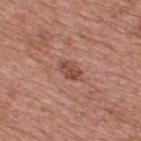Acquisition and patient details:
Longest diameter approximately 3 mm. A 15 mm crop from a total-body photograph taken for skin-cancer surveillance. The subject is a male about 70 years old. On the upper back. The total-body-photography lesion software estimated a lesion color around L≈48 a*≈24 b*≈27 in CIELAB, about 10 CIELAB-L* units darker than the surrounding skin, and a lesion-to-skin contrast of about 7 (normalized; higher = more distinct). And it measured a border-irregularity rating of about 2/10, internal color variation of about 2.5 on a 0–10 scale, and peripheral color asymmetry of about 1. The software also gave a classifier nevus-likeness of about 5/100. The tile uses white-light illumination.